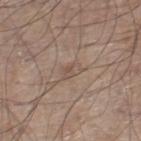Q: Was this lesion biopsied?
A: total-body-photography surveillance lesion; no biopsy